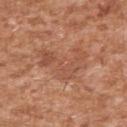This lesion was catalogued during total-body skin photography and was not selected for biopsy.
The subject is a male roughly 45 years of age.
About 5.5 mm across.
Captured under white-light illumination.
The lesion is on the right upper arm.
An algorithmic analysis of the crop reported a lesion–skin lightness drop of about 7. And it measured a border-irregularity rating of about 8.5/10, a color-variation rating of about 3.5/10, and radial color variation of about 1.5. It also reported a nevus-likeness score of about 0/100 and a detector confidence of about 100 out of 100 that the crop contains a lesion.
This image is a 15 mm lesion crop taken from a total-body photograph.